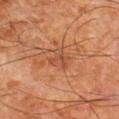The lesion was tiled from a total-body skin photograph and was not biopsied.